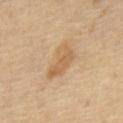Captured during whole-body skin photography for melanoma surveillance; the lesion was not biopsied. An algorithmic analysis of the crop reported a border-irregularity index near 4.5/10, a color-variation rating of about 3/10, and peripheral color asymmetry of about 1. And it measured an automated nevus-likeness rating near 10 out of 100. The tile uses cross-polarized illumination. A region of skin cropped from a whole-body photographic capture, roughly 15 mm wide. Measured at roughly 5 mm in maximum diameter. A male subject, aged approximately 65.